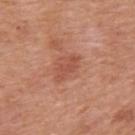Q: Was a biopsy performed?
A: imaged on a skin check; not biopsied
Q: Patient demographics?
A: male, in their 70s
Q: How was this image acquired?
A: ~15 mm crop, total-body skin-cancer survey
Q: Lesion location?
A: the back
Q: How large is the lesion?
A: about 3.5 mm